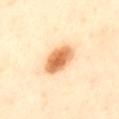Q: Was a biopsy performed?
A: imaged on a skin check; not biopsied